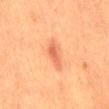biopsy status: no biopsy performed (imaged during a skin exam) | size: about 3.5 mm | site: the back | acquisition: 15 mm crop, total-body photography | subject: male, approximately 40 years of age | automated lesion analysis: an eccentricity of roughly 0.9 and two-axis asymmetry of about 0.35; a lesion color around L≈53 a*≈28 b*≈35 in CIELAB, about 9 CIELAB-L* units darker than the surrounding skin, and a lesion-to-skin contrast of about 6.5 (normalized; higher = more distinct); border irregularity of about 4 on a 0–10 scale | illumination: cross-polarized illumination.